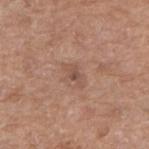Part of a total-body skin-imaging series; this lesion was reviewed on a skin check and was not flagged for biopsy.
The lesion is on the right upper arm.
A male subject about 65 years old.
A lesion tile, about 15 mm wide, cut from a 3D total-body photograph.
An algorithmic analysis of the crop reported a footprint of about 3 mm², an eccentricity of roughly 0.85, and two-axis asymmetry of about 0.4. The analysis additionally found an automated nevus-likeness rating near 0 out of 100 and a detector confidence of about 100 out of 100 that the crop contains a lesion.
Longest diameter approximately 3 mm.
Captured under white-light illumination.